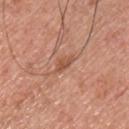Findings:
• workup · no biopsy performed (imaged during a skin exam)
• illumination · white-light illumination
• automated lesion analysis · an area of roughly 2.5 mm², an eccentricity of roughly 0.9, and a shape-asymmetry score of about 0.45 (0 = symmetric); a normalized lesion–skin contrast near 6.5; border irregularity of about 4.5 on a 0–10 scale, a within-lesion color-variation index near 0.5/10, and a peripheral color-asymmetry measure near 0
• subject · male, aged 38 to 42
• location · the chest
• acquisition · ~15 mm crop, total-body skin-cancer survey